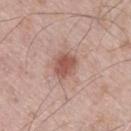Recorded during total-body skin imaging; not selected for excision or biopsy.
Cropped from a whole-body photographic skin survey; the tile spans about 15 mm.
The recorded lesion diameter is about 3 mm.
The subject is a male roughly 70 years of age.
From the leg.
Imaged with white-light lighting.